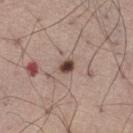Clinical impression:
Imaged during a routine full-body skin examination; the lesion was not biopsied and no histopathology is available.
Image and clinical context:
The lesion is located on the right thigh. A close-up tile cropped from a whole-body skin photograph, about 15 mm across. The patient is a male about 55 years old.